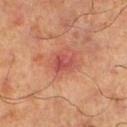Clinical impression: This lesion was catalogued during total-body skin photography and was not selected for biopsy. Image and clinical context: The recorded lesion diameter is about 2.5 mm. Imaged with cross-polarized lighting. A male subject, in their mid- to late 60s. A 15 mm close-up extracted from a 3D total-body photography capture. On the right lower leg.